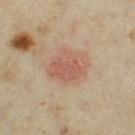  biopsy_status: not biopsied; imaged during a skin examination
  lesion_size:
    long_diameter_mm_approx: 4.0
  image:
    source: total-body photography crop
    field_of_view_mm: 15
  patient:
    sex: female
    age_approx: 35
  lighting: cross-polarized
  site: left thigh